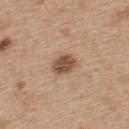Imaged during a routine full-body skin examination; the lesion was not biopsied and no histopathology is available. Automated image analysis of the tile measured a lesion area of about 6 mm², an outline eccentricity of about 0.6 (0 = round, 1 = elongated), and a shape-asymmetry score of about 0.15 (0 = symmetric). And it measured a border-irregularity index near 1.5/10, internal color variation of about 3.5 on a 0–10 scale, and radial color variation of about 1. And it measured a detector confidence of about 100 out of 100 that the crop contains a lesion. Measured at roughly 3 mm in maximum diameter. The lesion is on the upper back. Imaged with white-light lighting. A region of skin cropped from a whole-body photographic capture, roughly 15 mm wide. A female patient, aged 43–47.